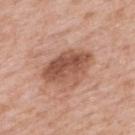| key | value |
|---|---|
| follow-up | imaged on a skin check; not biopsied |
| imaging modality | total-body-photography crop, ~15 mm field of view |
| lighting | white-light |
| location | the back |
| patient | female, aged 58–62 |